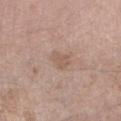Assessment: Captured during whole-body skin photography for melanoma surveillance; the lesion was not biopsied. Background: Located on the right forearm. The subject is a male roughly 60 years of age. Cropped from a whole-body photographic skin survey; the tile spans about 15 mm. The tile uses white-light illumination.